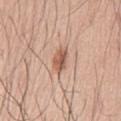follow-up: catalogued during a skin exam; not biopsied
automated lesion analysis: a footprint of about 5 mm² and a shape-asymmetry score of about 0.2 (0 = symmetric); a lesion color around L≈58 a*≈21 b*≈30 in CIELAB and a normalized lesion–skin contrast near 8
acquisition: 15 mm crop, total-body photography
lighting: white-light illumination
lesion size: ~3.5 mm (longest diameter)
patient: male, aged around 75
site: the chest